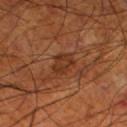- notes: imaged on a skin check; not biopsied
- image: ~15 mm crop, total-body skin-cancer survey
- tile lighting: cross-polarized illumination
- site: the left lower leg
- subject: male, aged 68–72